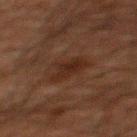The lesion was tiled from a total-body skin photograph and was not biopsied. A male patient, aged around 60. Located on the upper back. A 15 mm close-up extracted from a 3D total-body photography capture.